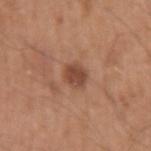Clinical impression:
The lesion was tiled from a total-body skin photograph and was not biopsied.
Image and clinical context:
The patient is a male about 50 years old. Captured under white-light illumination. The total-body-photography lesion software estimated a mean CIELAB color near L≈46 a*≈23 b*≈29, roughly 11 lightness units darker than nearby skin, and a normalized lesion–skin contrast near 8.5. The software also gave a classifier nevus-likeness of about 65/100 and lesion-presence confidence of about 100/100. This image is a 15 mm lesion crop taken from a total-body photograph. The lesion is on the left upper arm.